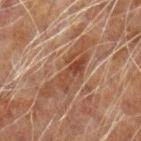The lesion was photographed on a routine skin check and not biopsied; there is no pathology result.
From the left forearm.
Longest diameter approximately 8 mm.
A lesion tile, about 15 mm wide, cut from a 3D total-body photograph.
Captured under cross-polarized illumination.
A male patient approximately 60 years of age.